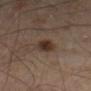Impression:
No biopsy was performed on this lesion — it was imaged during a full skin examination and was not determined to be concerning.
Image and clinical context:
Imaged with cross-polarized lighting. The lesion-visualizer software estimated a footprint of about 5 mm² and an eccentricity of roughly 0.8. It also reported a nevus-likeness score of about 95/100. Approximately 3 mm at its widest. A lesion tile, about 15 mm wide, cut from a 3D total-body photograph. Located on the leg. A male subject, in their 50s.